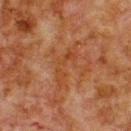<record>
  <biopsy_status>not biopsied; imaged during a skin examination</biopsy_status>
  <image>
    <source>total-body photography crop</source>
    <field_of_view_mm>15</field_of_view_mm>
  </image>
  <lesion_size>
    <long_diameter_mm_approx>5.5</long_diameter_mm_approx>
  </lesion_size>
  <patient>
    <sex>male</sex>
    <age_approx>80</age_approx>
  </patient>
  <site>upper back</site>
</record>